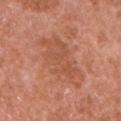patient:
  sex: male
  age_approx: 65
lesion_size:
  long_diameter_mm_approx: 8.0
site: left upper arm
image:
  source: total-body photography crop
  field_of_view_mm: 15